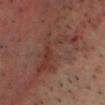<tbp_lesion>
  <biopsy_status>not biopsied; imaged during a skin examination</biopsy_status>
  <image>
    <source>total-body photography crop</source>
    <field_of_view_mm>15</field_of_view_mm>
  </image>
  <lesion_size>
    <long_diameter_mm_approx>7.0</long_diameter_mm_approx>
  </lesion_size>
  <lighting>cross-polarized</lighting>
  <patient>
    <sex>male</sex>
    <age_approx>55</age_approx>
  </patient>
  <site>head or neck</site>
</tbp_lesion>